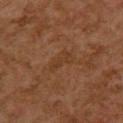Impression:
Recorded during total-body skin imaging; not selected for excision or biopsy.
Background:
An algorithmic analysis of the crop reported a footprint of about 4.5 mm², an eccentricity of roughly 0.9, and two-axis asymmetry of about 0.35. The analysis additionally found a lesion–skin lightness drop of about 4 and a lesion-to-skin contrast of about 5 (normalized; higher = more distinct). The analysis additionally found an automated nevus-likeness rating near 0 out of 100. On the upper back. The lesion's longest dimension is about 3.5 mm. A female subject aged 58 to 62. Captured under cross-polarized illumination. A roughly 15 mm field-of-view crop from a total-body skin photograph.